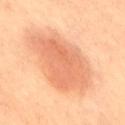Findings:
• biopsy status — catalogued during a skin exam; not biopsied
• image — ~15 mm tile from a whole-body skin photo
• site — the right thigh
• patient — roughly 60 years of age